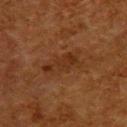subject = female, aged 48 to 52
size = about 5 mm
acquisition = ~15 mm tile from a whole-body skin photo
illumination = cross-polarized illumination
automated lesion analysis = a lesion color around L≈25 a*≈18 b*≈27 in CIELAB, about 5 CIELAB-L* units darker than the surrounding skin, and a normalized border contrast of about 6; a within-lesion color-variation index near 2/10 and peripheral color asymmetry of about 0.5; an automated nevus-likeness rating near 0 out of 100
anatomic site = the back